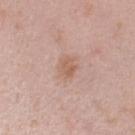Captured under white-light illumination. A 15 mm crop from a total-body photograph taken for skin-cancer surveillance. An algorithmic analysis of the crop reported a border-irregularity index near 2.5/10, a color-variation rating of about 2/10, and radial color variation of about 1. A male patient, roughly 40 years of age. On the left upper arm.